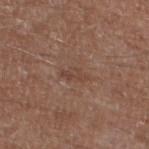biopsy_status: not biopsied; imaged during a skin examination
image:
  source: total-body photography crop
  field_of_view_mm: 15
lesion_size:
  long_diameter_mm_approx: 3.0
patient:
  sex: male
  age_approx: 65
lighting: white-light
site: left lower leg